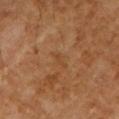notes: catalogued during a skin exam; not biopsied
image source: total-body-photography crop, ~15 mm field of view
anatomic site: the left upper arm
subject: male, aged around 55
lesion diameter: about 2.5 mm
tile lighting: cross-polarized illumination